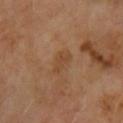Clinical impression:
Imaged during a routine full-body skin examination; the lesion was not biopsied and no histopathology is available.
Context:
From the chest. Cropped from a total-body skin-imaging series; the visible field is about 15 mm. A male patient, roughly 65 years of age.